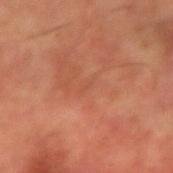Assessment:
Part of a total-body skin-imaging series; this lesion was reviewed on a skin check and was not flagged for biopsy.
Acquisition and patient details:
The lesion-visualizer software estimated internal color variation of about 0 on a 0–10 scale and radial color variation of about 0. A lesion tile, about 15 mm wide, cut from a 3D total-body photograph. On the arm. Captured under cross-polarized illumination. A male subject, roughly 60 years of age. About 1.5 mm across.